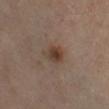Clinical impression:
Recorded during total-body skin imaging; not selected for excision or biopsy.
Background:
A male patient, aged around 65. Cropped from a total-body skin-imaging series; the visible field is about 15 mm. The lesion is located on the right lower leg. This is a cross-polarized tile.